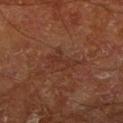Impression:
The lesion was photographed on a routine skin check and not biopsied; there is no pathology result.
Acquisition and patient details:
From the right lower leg. A 15 mm crop from a total-body photograph taken for skin-cancer surveillance. A male subject, aged around 65. Imaged with cross-polarized lighting. The total-body-photography lesion software estimated a detector confidence of about 100 out of 100 that the crop contains a lesion. The lesion's longest dimension is about 4.5 mm.